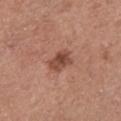Imaged during a routine full-body skin examination; the lesion was not biopsied and no histopathology is available. This is a white-light tile. Automated tile analysis of the lesion measured a mean CIELAB color near L≈47 a*≈23 b*≈28, about 11 CIELAB-L* units darker than the surrounding skin, and a normalized lesion–skin contrast near 8. It also reported a within-lesion color-variation index near 4.5/10. It also reported an automated nevus-likeness rating near 40 out of 100 and a lesion-detection confidence of about 100/100. A 15 mm close-up extracted from a 3D total-body photography capture. About 3 mm across. A female subject, in their 60s. From the front of the torso.